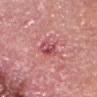No biopsy was performed on this lesion — it was imaged during a full skin examination and was not determined to be concerning.
The lesion is located on the head or neck.
Imaged with white-light lighting.
The lesion-visualizer software estimated a mean CIELAB color near L≈52 a*≈34 b*≈21, a lesion–skin lightness drop of about 10, and a normalized lesion–skin contrast near 7. And it measured a classifier nevus-likeness of about 0/100 and lesion-presence confidence of about 100/100.
A male patient, in their mid- to late 60s.
The lesion's longest dimension is about 2.5 mm.
A region of skin cropped from a whole-body photographic capture, roughly 15 mm wide.